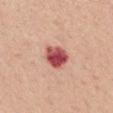biopsy status = no biopsy performed (imaged during a skin exam) | diameter = about 3.5 mm | subject = female, aged 53 to 57 | acquisition = total-body-photography crop, ~15 mm field of view | anatomic site = the mid back | illumination = white-light.